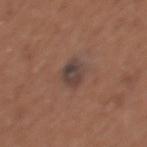Recorded during total-body skin imaging; not selected for excision or biopsy. Cropped from a whole-body photographic skin survey; the tile spans about 15 mm. A female patient, roughly 50 years of age. On the back. Approximately 3 mm at its widest. Captured under white-light illumination.